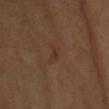No biopsy was performed on this lesion — it was imaged during a full skin examination and was not determined to be concerning. The total-body-photography lesion software estimated a lesion area of about 2.5 mm², a shape eccentricity near 0.9, and two-axis asymmetry of about 0.5. It also reported a mean CIELAB color near L≈33 a*≈17 b*≈28, roughly 5 lightness units darker than nearby skin, and a normalized lesion–skin contrast near 5. The lesion's longest dimension is about 2.5 mm. A 15 mm crop from a total-body photograph taken for skin-cancer surveillance. A female patient aged 53 to 57. From the left forearm.